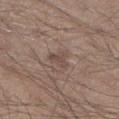Impression: Recorded during total-body skin imaging; not selected for excision or biopsy. Image and clinical context: Cropped from a total-body skin-imaging series; the visible field is about 15 mm. An algorithmic analysis of the crop reported a footprint of about 4 mm², a shape eccentricity near 0.55, and two-axis asymmetry of about 0.5. And it measured a lesion–skin lightness drop of about 7 and a normalized lesion–skin contrast near 5.5. The software also gave a border-irregularity index near 5/10, internal color variation of about 2 on a 0–10 scale, and a peripheral color-asymmetry measure near 1. And it measured a detector confidence of about 100 out of 100 that the crop contains a lesion. Located on the right lower leg. The recorded lesion diameter is about 3 mm. A male subject, aged 28 to 32.